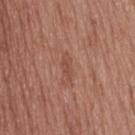Recorded during total-body skin imaging; not selected for excision or biopsy.
The lesion is on the upper back.
Automated tile analysis of the lesion measured a footprint of about 3 mm², an outline eccentricity of about 0.95 (0 = round, 1 = elongated), and a symmetry-axis asymmetry near 0.25. The software also gave a nevus-likeness score of about 0/100 and lesion-presence confidence of about 100/100.
Longest diameter approximately 3.5 mm.
The tile uses white-light illumination.
The subject is a male roughly 40 years of age.
A region of skin cropped from a whole-body photographic capture, roughly 15 mm wide.